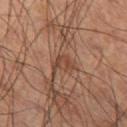Imaged during a routine full-body skin examination; the lesion was not biopsied and no histopathology is available. The lesion's longest dimension is about 2.5 mm. A male subject aged around 60. A lesion tile, about 15 mm wide, cut from a 3D total-body photograph. The lesion-visualizer software estimated a lesion area of about 4 mm² and a shape eccentricity near 0.7. The analysis additionally found a lesion color around L≈42 a*≈20 b*≈28 in CIELAB and roughly 8 lightness units darker than nearby skin. And it measured a classifier nevus-likeness of about 0/100. Imaged with cross-polarized lighting. The lesion is located on the left thigh.